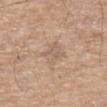- biopsy status: no biopsy performed (imaged during a skin exam)